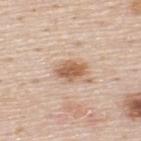The lesion was photographed on a routine skin check and not biopsied; there is no pathology result.
This is a white-light tile.
On the back.
A male patient in their mid- to late 40s.
The recorded lesion diameter is about 3.5 mm.
Cropped from a whole-body photographic skin survey; the tile spans about 15 mm.